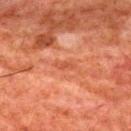Case summary:
- workup: catalogued during a skin exam; not biopsied
- acquisition: ~15 mm crop, total-body skin-cancer survey
- patient: male, aged approximately 80
- illumination: cross-polarized
- anatomic site: the mid back
- diameter: about 2.5 mm
- automated lesion analysis: a mean CIELAB color near L≈43 a*≈26 b*≈32, about 6 CIELAB-L* units darker than the surrounding skin, and a normalized border contrast of about 5